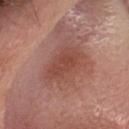No biopsy was performed on this lesion — it was imaged during a full skin examination and was not determined to be concerning.
About 5 mm across.
Imaged with white-light lighting.
On the head or neck.
A male subject aged 28 to 32.
A 15 mm crop from a total-body photograph taken for skin-cancer surveillance.
The total-body-photography lesion software estimated a lesion color around L≈47 a*≈24 b*≈27 in CIELAB, about 8 CIELAB-L* units darker than the surrounding skin, and a normalized lesion–skin contrast near 6.5. And it measured an automated nevus-likeness rating near 15 out of 100 and a detector confidence of about 100 out of 100 that the crop contains a lesion.